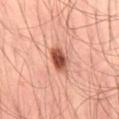Clinical impression: The lesion was photographed on a routine skin check and not biopsied; there is no pathology result. Context: The tile uses cross-polarized illumination. Automated image analysis of the tile measured a footprint of about 7 mm², an eccentricity of roughly 0.8, and a shape-asymmetry score of about 0.2 (0 = symmetric). The analysis additionally found a mean CIELAB color near L≈51 a*≈27 b*≈30, a lesion–skin lightness drop of about 16, and a lesion-to-skin contrast of about 10.5 (normalized; higher = more distinct). Located on the right thigh. A male patient, in their mid-40s. A region of skin cropped from a whole-body photographic capture, roughly 15 mm wide. Longest diameter approximately 4 mm.